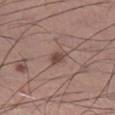Recorded during total-body skin imaging; not selected for excision or biopsy. A 15 mm crop from a total-body photograph taken for skin-cancer surveillance. Longest diameter approximately 3 mm. On the right lower leg. A male subject aged approximately 60. This is a white-light tile. An algorithmic analysis of the crop reported a lesion area of about 3 mm², an eccentricity of roughly 0.85, and a shape-asymmetry score of about 0.25 (0 = symmetric). And it measured a border-irregularity index near 2.5/10, a within-lesion color-variation index near 2/10, and a peripheral color-asymmetry measure near 0.5.